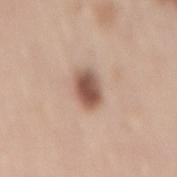Q: Was this lesion biopsied?
A: catalogued during a skin exam; not biopsied
Q: What kind of image is this?
A: ~15 mm tile from a whole-body skin photo
Q: Illumination type?
A: white-light illumination
Q: Who is the patient?
A: female, about 50 years old
Q: Lesion location?
A: the mid back
Q: What is the lesion's diameter?
A: ~4 mm (longest diameter)